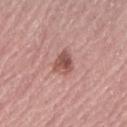biopsy_status: not biopsied; imaged during a skin examination
site: right upper arm
patient:
  sex: female
  age_approx: 65
image:
  source: total-body photography crop
  field_of_view_mm: 15
lighting: white-light